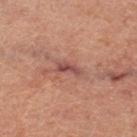image-analysis metrics: a lesion–skin lightness drop of about 10 and a normalized border contrast of about 8; border irregularity of about 3 on a 0–10 scale, a within-lesion color-variation index near 1/10, and peripheral color asymmetry of about 0.5; a nevus-likeness score of about 0/100 and lesion-presence confidence of about 80/100 | imaging modality: ~15 mm tile from a whole-body skin photo | diameter: ~3 mm (longest diameter) | patient: male, aged 58 to 62 | anatomic site: the leg | lighting: cross-polarized illumination.